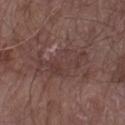Impression: The lesion was photographed on a routine skin check and not biopsied; there is no pathology result. Image and clinical context: From the arm. A 15 mm crop from a total-body photograph taken for skin-cancer surveillance. The subject is a male aged around 65.